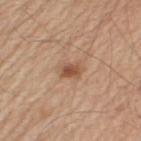Imaged during a routine full-body skin examination; the lesion was not biopsied and no histopathology is available. The lesion-visualizer software estimated roughly 11 lightness units darker than nearby skin and a normalized lesion–skin contrast near 7.5. It also reported a border-irregularity index near 2/10, internal color variation of about 3.5 on a 0–10 scale, and peripheral color asymmetry of about 1. And it measured an automated nevus-likeness rating near 80 out of 100 and a detector confidence of about 100 out of 100 that the crop contains a lesion. From the left upper arm. Captured under white-light illumination. A male patient approximately 65 years of age. A lesion tile, about 15 mm wide, cut from a 3D total-body photograph. Approximately 2.5 mm at its widest.